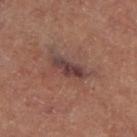biopsy status: no biopsy performed (imaged during a skin exam)
image: ~15 mm crop, total-body skin-cancer survey
subject: female
site: the left thigh